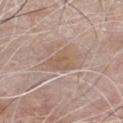Part of a total-body skin-imaging series; this lesion was reviewed on a skin check and was not flagged for biopsy.